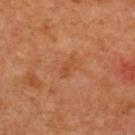Q: Was this lesion biopsied?
A: imaged on a skin check; not biopsied
Q: Who is the patient?
A: female, roughly 40 years of age
Q: Automated lesion metrics?
A: an area of roughly 3 mm²; a lesion color around L≈50 a*≈27 b*≈39 in CIELAB and a normalized lesion–skin contrast near 5; a nevus-likeness score of about 0/100 and a lesion-detection confidence of about 100/100
Q: How was this image acquired?
A: 15 mm crop, total-body photography
Q: Where on the body is the lesion?
A: the back